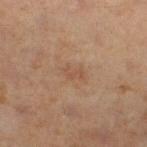{"biopsy_status": "not biopsied; imaged during a skin examination", "patient": {"sex": "male", "age_approx": 60}, "site": "right lower leg", "lighting": "cross-polarized", "automated_metrics": {"area_mm2_approx": 3.0, "shape_asymmetry": 0.45, "cielab_L": 47, "cielab_a": 18, "cielab_b": 29, "vs_skin_darker_L": 6.0, "vs_skin_contrast_norm": 4.5, "nevus_likeness_0_100": 0, "lesion_detection_confidence_0_100": 100}, "lesion_size": {"long_diameter_mm_approx": 2.5}, "image": {"source": "total-body photography crop", "field_of_view_mm": 15}}